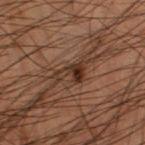Captured during whole-body skin photography for melanoma surveillance; the lesion was not biopsied.
The tile uses cross-polarized illumination.
A male subject aged 58–62.
A 15 mm close-up extracted from a 3D total-body photography capture.
The lesion is on the left thigh.
Approximately 3.5 mm at its widest.